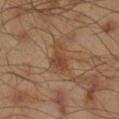The lesion was tiled from a total-body skin photograph and was not biopsied.
Captured under cross-polarized illumination.
Cropped from a total-body skin-imaging series; the visible field is about 15 mm.
The total-body-photography lesion software estimated a border-irregularity rating of about 3.5/10 and internal color variation of about 3 on a 0–10 scale. It also reported a classifier nevus-likeness of about 5/100 and a lesion-detection confidence of about 100/100.
The lesion is located on the right lower leg.
Longest diameter approximately 3.5 mm.
The subject is a male aged approximately 45.